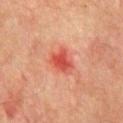<record>
  <biopsy_status>not biopsied; imaged during a skin examination</biopsy_status>
  <patient>
    <sex>male</sex>
    <age_approx>75</age_approx>
  </patient>
  <site>chest</site>
  <image>
    <source>total-body photography crop</source>
    <field_of_view_mm>15</field_of_view_mm>
  </image>
</record>